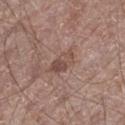biopsy status: no biopsy performed (imaged during a skin exam)
lighting: white-light illumination
location: the left thigh
lesion size: ~4 mm (longest diameter)
patient: male, aged 68–72
TBP lesion metrics: a lesion area of about 5.5 mm², an outline eccentricity of about 0.85 (0 = round, 1 = elongated), and a shape-asymmetry score of about 0.35 (0 = symmetric); an average lesion color of about L≈48 a*≈18 b*≈24 (CIELAB), roughly 8 lightness units darker than nearby skin, and a lesion-to-skin contrast of about 6.5 (normalized; higher = more distinct); lesion-presence confidence of about 100/100
acquisition: ~15 mm tile from a whole-body skin photo